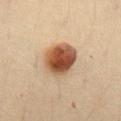Clinical impression: Imaged during a routine full-body skin examination; the lesion was not biopsied and no histopathology is available. Acquisition and patient details: The lesion is on the front of the torso. About 4 mm across. A female patient, aged 33 to 37. Cropped from a whole-body photographic skin survey; the tile spans about 15 mm. Imaged with cross-polarized lighting.